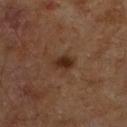{
  "biopsy_status": "not biopsied; imaged during a skin examination",
  "site": "leg",
  "patient": {
    "sex": "male",
    "age_approx": 60
  },
  "automated_metrics": {
    "cielab_L": 26,
    "cielab_a": 17,
    "cielab_b": 25,
    "vs_skin_darker_L": 9.0,
    "vs_skin_contrast_norm": 9.5
  },
  "lesion_size": {
    "long_diameter_mm_approx": 2.5
  },
  "image": {
    "source": "total-body photography crop",
    "field_of_view_mm": 15
  }
}